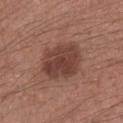* follow-up — no biopsy performed (imaged during a skin exam)
* image source — ~15 mm tile from a whole-body skin photo
* body site — the left forearm
* patient — male, in their mid-30s
* size — about 5.5 mm